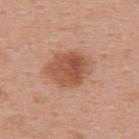biopsy_status: not biopsied; imaged during a skin examination
image:
  source: total-body photography crop
  field_of_view_mm: 15
lesion_size:
  long_diameter_mm_approx: 5.5
site: back
automated_metrics:
  area_mm2_approx: 16.0
  eccentricity: 0.6
  shape_asymmetry: 0.15
  cielab_L: 53
  cielab_a: 24
  cielab_b: 32
  border_irregularity_0_10: 2.0
  color_variation_0_10: 4.5
  nevus_likeness_0_100: 75
  lesion_detection_confidence_0_100: 100
patient:
  sex: female
  age_approx: 50